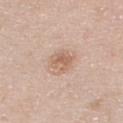Case summary:
- workup: no biopsy performed (imaged during a skin exam)
- image-analysis metrics: an automated nevus-likeness rating near 35 out of 100 and lesion-presence confidence of about 100/100
- location: the upper back
- acquisition: total-body-photography crop, ~15 mm field of view
- lesion size: about 2.5 mm
- patient: male, approximately 40 years of age